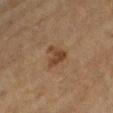Q: Was a biopsy performed?
A: no biopsy performed (imaged during a skin exam)
Q: How was the tile lit?
A: cross-polarized illumination
Q: How was this image acquired?
A: ~15 mm tile from a whole-body skin photo
Q: Where on the body is the lesion?
A: the leg
Q: What are the patient's age and sex?
A: male, aged around 85 or older
Q: Lesion size?
A: ~3 mm (longest diameter)